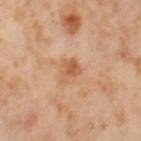Recorded during total-body skin imaging; not selected for excision or biopsy.
Cropped from a total-body skin-imaging series; the visible field is about 15 mm.
The total-body-photography lesion software estimated an eccentricity of roughly 0.65. The software also gave a mean CIELAB color near L≈59 a*≈22 b*≈37, about 9 CIELAB-L* units darker than the surrounding skin, and a lesion-to-skin contrast of about 6.5 (normalized; higher = more distinct). It also reported border irregularity of about 3.5 on a 0–10 scale, a within-lesion color-variation index near 3.5/10, and a peripheral color-asymmetry measure near 1. It also reported an automated nevus-likeness rating near 0 out of 100.
The patient is a female approximately 55 years of age.
The lesion is on the left thigh.
Measured at roughly 3 mm in maximum diameter.
Captured under cross-polarized illumination.